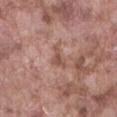Q: Lesion location?
A: the abdomen
Q: What is the imaging modality?
A: ~15 mm crop, total-body skin-cancer survey
Q: Patient demographics?
A: male, in their mid- to late 70s
Q: What lighting was used for the tile?
A: white-light
Q: How large is the lesion?
A: about 3 mm